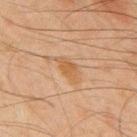automated lesion analysis: an average lesion color of about L≈47 a*≈18 b*≈32 (CIELAB), a lesion–skin lightness drop of about 7, and a normalized lesion–skin contrast near 6.5; a border-irregularity index near 2.5/10, a within-lesion color-variation index near 1.5/10, and radial color variation of about 0.5
acquisition: ~15 mm tile from a whole-body skin photo
site: the mid back
patient: male, aged 43 to 47
tile lighting: cross-polarized illumination
lesion diameter: ≈3 mm